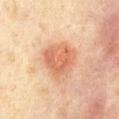Notes:
– follow-up: no biopsy performed (imaged during a skin exam)
– patient: male, aged 58 to 62
– anatomic site: the front of the torso
– acquisition: ~15 mm tile from a whole-body skin photo